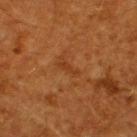Case summary:
* automated lesion analysis · a lesion area of about 2 mm² and a shape eccentricity near 0.95; a lesion color around L≈35 a*≈24 b*≈37 in CIELAB, a lesion–skin lightness drop of about 6, and a normalized lesion–skin contrast near 5.5; a nevus-likeness score of about 0/100 and lesion-presence confidence of about 100/100
* patient · male, aged around 60
* body site · the upper back
* image · ~15 mm crop, total-body skin-cancer survey
* tile lighting · cross-polarized illumination
* size · about 2.5 mm